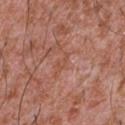The lesion was tiled from a total-body skin photograph and was not biopsied.
Automated tile analysis of the lesion measured border irregularity of about 5.5 on a 0–10 scale, a within-lesion color-variation index near 0/10, and radial color variation of about 0.
The lesion is located on the chest.
The tile uses white-light illumination.
A lesion tile, about 15 mm wide, cut from a 3D total-body photograph.
Measured at roughly 2.5 mm in maximum diameter.
A male subject in their mid- to late 40s.